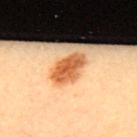Clinical impression:
The lesion was photographed on a routine skin check and not biopsied; there is no pathology result.
Context:
This is a cross-polarized tile. The recorded lesion diameter is about 4.5 mm. A region of skin cropped from a whole-body photographic capture, roughly 15 mm wide. A female patient aged 33–37. Automated image analysis of the tile measured radial color variation of about 1.5. It also reported a detector confidence of about 100 out of 100 that the crop contains a lesion. On the upper back.